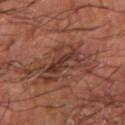Part of a total-body skin-imaging series; this lesion was reviewed on a skin check and was not flagged for biopsy. The lesion's longest dimension is about 4 mm. From the arm. The lesion-visualizer software estimated a footprint of about 6 mm² and a shape-asymmetry score of about 0.4 (0 = symmetric). It also reported an automated nevus-likeness rating near 0 out of 100 and a detector confidence of about 80 out of 100 that the crop contains a lesion. A patient aged approximately 65. Imaged with cross-polarized lighting. A 15 mm close-up extracted from a 3D total-body photography capture.